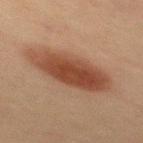No biopsy was performed on this lesion — it was imaged during a full skin examination and was not determined to be concerning.
A male patient aged around 60.
Located on the back.
Cropped from a whole-body photographic skin survey; the tile spans about 15 mm.